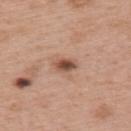biopsy_status: not biopsied; imaged during a skin examination
lesion_size:
  long_diameter_mm_approx: 2.5
site: upper back
image:
  source: total-body photography crop
  field_of_view_mm: 15
patient:
  sex: male
  age_approx: 60